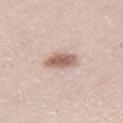The lesion was tiled from a total-body skin photograph and was not biopsied. The total-body-photography lesion software estimated roughly 14 lightness units darker than nearby skin and a lesion-to-skin contrast of about 9 (normalized; higher = more distinct). The software also gave a border-irregularity rating of about 2/10, a within-lesion color-variation index near 3/10, and peripheral color asymmetry of about 1. A female subject aged approximately 45. Approximately 4 mm at its widest. The lesion is located on the right thigh. A region of skin cropped from a whole-body photographic capture, roughly 15 mm wide.